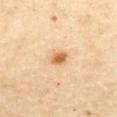No biopsy was performed on this lesion — it was imaged during a full skin examination and was not determined to be concerning.
Imaged with cross-polarized lighting.
About 2.5 mm across.
A roughly 15 mm field-of-view crop from a total-body skin photograph.
The patient is a male in their 70s.
Located on the abdomen.
Automated image analysis of the tile measured a footprint of about 4 mm², an eccentricity of roughly 0.7, and two-axis asymmetry of about 0.2.